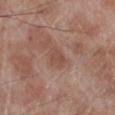biopsy status: total-body-photography surveillance lesion; no biopsy | diameter: ≈2.5 mm | location: the left lower leg | tile lighting: white-light illumination | image: 15 mm crop, total-body photography | subject: male, aged around 70.